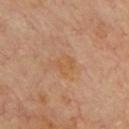Q: Is there a histopathology result?
A: no biopsy performed (imaged during a skin exam)
Q: How large is the lesion?
A: ≈3 mm
Q: What lighting was used for the tile?
A: cross-polarized
Q: What is the imaging modality?
A: ~15 mm crop, total-body skin-cancer survey
Q: What did automated image analysis measure?
A: an area of roughly 4.5 mm², an eccentricity of roughly 0.6, and a shape-asymmetry score of about 0.35 (0 = symmetric); a mean CIELAB color near L≈57 a*≈21 b*≈38, about 5 CIELAB-L* units darker than the surrounding skin, and a normalized border contrast of about 5.5; a border-irregularity index near 4/10 and internal color variation of about 2 on a 0–10 scale; a nevus-likeness score of about 0/100
Q: What is the anatomic site?
A: the front of the torso